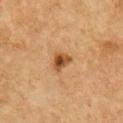notes — catalogued during a skin exam; not biopsied | lesion diameter — ≈3 mm | acquisition — 15 mm crop, total-body photography | subject — male, aged 73 to 77 | lighting — cross-polarized illumination | location — the chest.